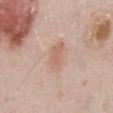workup: catalogued during a skin exam; not biopsied | anatomic site: the abdomen | acquisition: ~15 mm tile from a whole-body skin photo | subject: male, aged around 50.